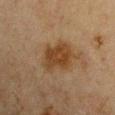Assessment:
Part of a total-body skin-imaging series; this lesion was reviewed on a skin check and was not flagged for biopsy.